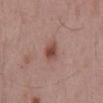<case>
  <biopsy_status>not biopsied; imaged during a skin examination</biopsy_status>
  <site>mid back</site>
  <lighting>white-light</lighting>
  <patient>
    <sex>male</sex>
    <age_approx>55</age_approx>
  </patient>
  <automated_metrics>
    <eccentricity>0.7</eccentricity>
    <shape_asymmetry>0.2</shape_asymmetry>
    <vs_skin_darker_L>11.0</vs_skin_darker_L>
    <vs_skin_contrast_norm>8.0</vs_skin_contrast_norm>
    <border_irregularity_0_10>2.0</border_irregularity_0_10>
    <color_variation_0_10>3.5</color_variation_0_10>
    <peripheral_color_asymmetry>1.0</peripheral_color_asymmetry>
    <nevus_likeness_0_100>90</nevus_likeness_0_100>
    <lesion_detection_confidence_0_100>100</lesion_detection_confidence_0_100>
  </automated_metrics>
  <lesion_size>
    <long_diameter_mm_approx>2.5</long_diameter_mm_approx>
  </lesion_size>
  <image>
    <source>total-body photography crop</source>
    <field_of_view_mm>15</field_of_view_mm>
  </image>
</case>